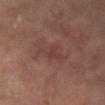Assessment: The lesion was tiled from a total-body skin photograph and was not biopsied. Background: This is a cross-polarized tile. This image is a 15 mm lesion crop taken from a total-body photograph. On the right lower leg. The subject is a male in their mid-50s.